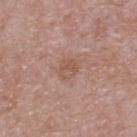This lesion was catalogued during total-body skin photography and was not selected for biopsy.
The lesion is on the upper back.
This is a white-light tile.
Cropped from a whole-body photographic skin survey; the tile spans about 15 mm.
A male subject, aged approximately 55.